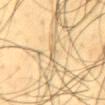Q: Was a biopsy performed?
A: total-body-photography surveillance lesion; no biopsy
Q: What is the imaging modality?
A: 15 mm crop, total-body photography
Q: Where on the body is the lesion?
A: the upper back
Q: Patient demographics?
A: male, roughly 45 years of age